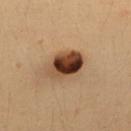Assessment:
This lesion was catalogued during total-body skin photography and was not selected for biopsy.
Background:
The patient is a female approximately 35 years of age. Captured under cross-polarized illumination. The lesion is located on the upper back. Cropped from a whole-body photographic skin survey; the tile spans about 15 mm. The lesion's longest dimension is about 4.5 mm.